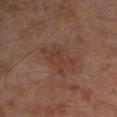Q: Is there a histopathology result?
A: total-body-photography surveillance lesion; no biopsy
Q: What lighting was used for the tile?
A: cross-polarized
Q: Automated lesion metrics?
A: a footprint of about 9.5 mm², an outline eccentricity of about 0.85 (0 = round, 1 = elongated), and a shape-asymmetry score of about 0.35 (0 = symmetric); a lesion color around L≈38 a*≈20 b*≈26 in CIELAB, a lesion–skin lightness drop of about 6, and a lesion-to-skin contrast of about 5.5 (normalized; higher = more distinct); internal color variation of about 2.5 on a 0–10 scale
Q: Lesion size?
A: about 5 mm
Q: What is the anatomic site?
A: the arm
Q: What are the patient's age and sex?
A: male, in their mid- to late 50s
Q: How was this image acquired?
A: 15 mm crop, total-body photography